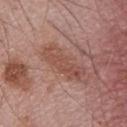– biopsy status: imaged on a skin check; not biopsied
– subject: male, aged approximately 75
– diameter: ~6 mm (longest diameter)
– tile lighting: white-light
– image source: 15 mm crop, total-body photography
– location: the chest